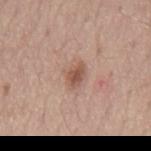biopsy status — total-body-photography surveillance lesion; no biopsy | lighting — white-light | subject — male, approximately 55 years of age | image — 15 mm crop, total-body photography | lesion diameter — ~2.5 mm (longest diameter) | automated lesion analysis — a lesion color around L≈54 a*≈20 b*≈27 in CIELAB, a lesion–skin lightness drop of about 11, and a normalized border contrast of about 7.5; an automated nevus-likeness rating near 35 out of 100 and a lesion-detection confidence of about 100/100 | location — the mid back.